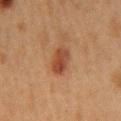Assessment: The lesion was photographed on a routine skin check and not biopsied; there is no pathology result. Image and clinical context: A 15 mm crop from a total-body photograph taken for skin-cancer surveillance. Located on the chest. A female patient roughly 40 years of age. Automated image analysis of the tile measured border irregularity of about 2 on a 0–10 scale and internal color variation of about 4 on a 0–10 scale.